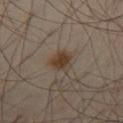Clinical impression:
The lesion was tiled from a total-body skin photograph and was not biopsied.
Acquisition and patient details:
This is a cross-polarized tile. Longest diameter approximately 2.5 mm. The total-body-photography lesion software estimated a lesion area of about 5.5 mm² and an eccentricity of roughly 0.5. The software also gave roughly 9 lightness units darker than nearby skin and a lesion-to-skin contrast of about 9.5 (normalized; higher = more distinct). And it measured a border-irregularity rating of about 2/10, a within-lesion color-variation index near 4/10, and peripheral color asymmetry of about 1.5. The analysis additionally found an automated nevus-likeness rating near 95 out of 100 and lesion-presence confidence of about 100/100. A 15 mm close-up extracted from a 3D total-body photography capture. Located on the leg. A male patient aged approximately 55.